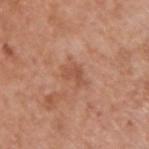Captured during whole-body skin photography for melanoma surveillance; the lesion was not biopsied. A roughly 15 mm field-of-view crop from a total-body skin photograph. A male subject, aged approximately 65. Approximately 3 mm at its widest. From the back. An algorithmic analysis of the crop reported an eccentricity of roughly 0.8 and two-axis asymmetry of about 0.4. It also reported a border-irregularity index near 4.5/10, a color-variation rating of about 1.5/10, and a peripheral color-asymmetry measure near 0.5. The tile uses white-light illumination.